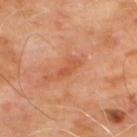This is a cross-polarized tile.
From the upper back.
The total-body-photography lesion software estimated an average lesion color of about L≈53 a*≈28 b*≈36 (CIELAB), about 8 CIELAB-L* units darker than the surrounding skin, and a lesion-to-skin contrast of about 5.5 (normalized; higher = more distinct). And it measured a color-variation rating of about 0.5/10. The analysis additionally found an automated nevus-likeness rating near 0 out of 100 and a detector confidence of about 100 out of 100 that the crop contains a lesion.
This image is a 15 mm lesion crop taken from a total-body photograph.
A male subject, approximately 65 years of age.